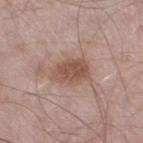The lesion was tiled from a total-body skin photograph and was not biopsied.
A lesion tile, about 15 mm wide, cut from a 3D total-body photograph.
Measured at roughly 4 mm in maximum diameter.
Located on the right thigh.
A male patient in their mid- to late 50s.
Imaged with white-light lighting.